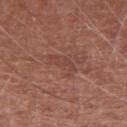follow-up: imaged on a skin check; not biopsied | subject: male, about 65 years old | anatomic site: the right upper arm | acquisition: total-body-photography crop, ~15 mm field of view.